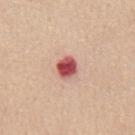Case summary:
- follow-up: catalogued during a skin exam; not biopsied